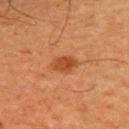Captured during whole-body skin photography for melanoma surveillance; the lesion was not biopsied. A male subject, aged 63–67. From the mid back. A 15 mm close-up tile from a total-body photography series done for melanoma screening.